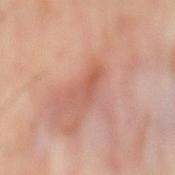Findings:
• follow-up · imaged on a skin check; not biopsied
• size · about 4 mm
• patient · male, approximately 50 years of age
• tile lighting · cross-polarized
• TBP lesion metrics · a mean CIELAB color near L≈56 a*≈25 b*≈30, roughly 7 lightness units darker than nearby skin, and a normalized lesion–skin contrast near 5.5; a nevus-likeness score of about 0/100 and lesion-presence confidence of about 85/100
• body site · the back
• imaging modality · ~15 mm tile from a whole-body skin photo